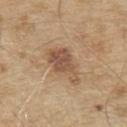Captured during whole-body skin photography for melanoma surveillance; the lesion was not biopsied. Automated tile analysis of the lesion measured a classifier nevus-likeness of about 30/100 and a lesion-detection confidence of about 100/100. Captured under white-light illumination. A 15 mm crop from a total-body photograph taken for skin-cancer surveillance. Located on the chest. A male subject, roughly 70 years of age. Longest diameter approximately 5.5 mm.